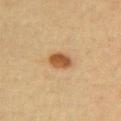The lesion was photographed on a routine skin check and not biopsied; there is no pathology result.
The subject is a female aged around 30.
The tile uses cross-polarized illumination.
Longest diameter approximately 3 mm.
On the chest.
A close-up tile cropped from a whole-body skin photograph, about 15 mm across.